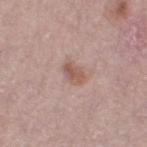acquisition: ~15 mm crop, total-body skin-cancer survey | subject: female, aged approximately 65 | image-analysis metrics: a shape eccentricity near 0.8 and two-axis asymmetry of about 0.25; about 10 CIELAB-L* units darker than the surrounding skin and a lesion-to-skin contrast of about 7 (normalized; higher = more distinct); a border-irregularity index near 2.5/10 and a peripheral color-asymmetry measure near 1; an automated nevus-likeness rating near 60 out of 100 and a lesion-detection confidence of about 100/100 | body site: the left thigh | diameter: ≈3 mm | tile lighting: white-light illumination.